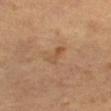Case summary:
* subject: female, aged 68 to 72
* anatomic site: the right thigh
* acquisition: total-body-photography crop, ~15 mm field of view
* illumination: cross-polarized
* size: ≈3 mm
* automated metrics: an area of roughly 3.5 mm², a shape eccentricity near 0.9, and a symmetry-axis asymmetry near 0.4; border irregularity of about 5 on a 0–10 scale, a color-variation rating of about 0/10, and a peripheral color-asymmetry measure near 0